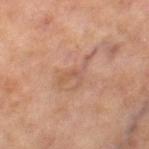Captured during whole-body skin photography for melanoma surveillance; the lesion was not biopsied.
Cropped from a total-body skin-imaging series; the visible field is about 15 mm.
From the leg.
A female patient approximately 60 years of age.